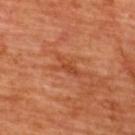Notes:
- biopsy status — total-body-photography surveillance lesion; no biopsy
- anatomic site — the upper back
- acquisition — 15 mm crop, total-body photography
- patient — male, approximately 65 years of age